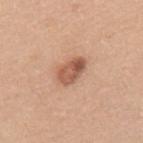Impression:
The lesion was photographed on a routine skin check and not biopsied; there is no pathology result.
Clinical summary:
A male patient, approximately 40 years of age. The recorded lesion diameter is about 3.5 mm. From the upper back. A 15 mm crop from a total-body photograph taken for skin-cancer surveillance. The total-body-photography lesion software estimated a lesion area of about 7 mm², a shape eccentricity near 0.8, and a shape-asymmetry score of about 0.25 (0 = symmetric). And it measured an average lesion color of about L≈56 a*≈23 b*≈31 (CIELAB), roughly 13 lightness units darker than nearby skin, and a normalized border contrast of about 8.5. The analysis additionally found a nevus-likeness score of about 80/100 and a lesion-detection confidence of about 100/100.